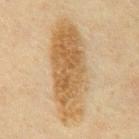follow-up — no biopsy performed (imaged during a skin exam) | site — the chest | illumination — cross-polarized illumination | subject — male, aged 58–62 | image source — 15 mm crop, total-body photography | size — about 12.5 mm.